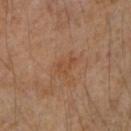biopsy status: total-body-photography surveillance lesion; no biopsy | lesion diameter: ≈3 mm | image-analysis metrics: an average lesion color of about L≈48 a*≈22 b*≈33 (CIELAB), about 5 CIELAB-L* units darker than the surrounding skin, and a normalized border contrast of about 5; a border-irregularity index near 4/10, a within-lesion color-variation index near 1.5/10, and a peripheral color-asymmetry measure near 0.5 | image: ~15 mm tile from a whole-body skin photo | subject: female, in their mid-40s | illumination: cross-polarized | location: the right upper arm.